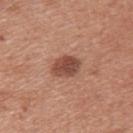{
  "biopsy_status": "not biopsied; imaged during a skin examination",
  "image": {
    "source": "total-body photography crop",
    "field_of_view_mm": 15
  },
  "automated_metrics": {
    "cielab_L": 48,
    "cielab_a": 23,
    "cielab_b": 28,
    "vs_skin_darker_L": 13.0,
    "vs_skin_contrast_norm": 9.0
  },
  "patient": {
    "sex": "male",
    "age_approx": 30
  },
  "site": "back"
}